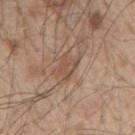Recorded during total-body skin imaging; not selected for excision or biopsy. A male subject, roughly 50 years of age. From the right upper arm. A lesion tile, about 15 mm wide, cut from a 3D total-body photograph.